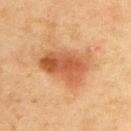  biopsy_status: not biopsied; imaged during a skin examination
  automated_metrics:
    area_mm2_approx: 18.0
    eccentricity: 0.8
    shape_asymmetry: 0.3
  lesion_size:
    long_diameter_mm_approx: 6.5
  lighting: cross-polarized
  site: back
  patient:
    sex: male
    age_approx: 45
  image:
    source: total-body photography crop
    field_of_view_mm: 15